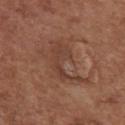Impression: No biopsy was performed on this lesion — it was imaged during a full skin examination and was not determined to be concerning. Image and clinical context: A region of skin cropped from a whole-body photographic capture, roughly 15 mm wide. The patient is a female roughly 75 years of age. On the chest.